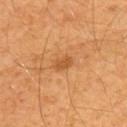Findings:
– workup: catalogued during a skin exam; not biopsied